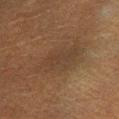Recorded during total-body skin imaging; not selected for excision or biopsy. A male subject aged 48 to 52. The total-body-photography lesion software estimated a footprint of about 15 mm² and an outline eccentricity of about 0.85 (0 = round, 1 = elongated). The analysis additionally found an average lesion color of about L≈30 a*≈13 b*≈23 (CIELAB), a lesion–skin lightness drop of about 5, and a normalized border contrast of about 5. The analysis additionally found a nevus-likeness score of about 0/100 and a detector confidence of about 90 out of 100 that the crop contains a lesion. A roughly 15 mm field-of-view crop from a total-body skin photograph. Longest diameter approximately 6.5 mm. The lesion is on the right lower leg. This is a cross-polarized tile.